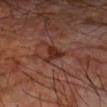<case>
  <biopsy_status>not biopsied; imaged during a skin examination</biopsy_status>
  <lesion_size>
    <long_diameter_mm_approx>2.5</long_diameter_mm_approx>
  </lesion_size>
  <patient>
    <sex>male</sex>
    <age_approx>70</age_approx>
  </patient>
  <site>right forearm</site>
  <lighting>cross-polarized</lighting>
  <automated_metrics>
    <area_mm2_approx>4.5</area_mm2_approx>
    <shape_asymmetry>0.55</shape_asymmetry>
    <border_irregularity_0_10>5.0</border_irregularity_0_10>
    <color_variation_0_10>2.5</color_variation_0_10>
    <peripheral_color_asymmetry>1.0</peripheral_color_asymmetry>
    <nevus_likeness_0_100>10</nevus_likeness_0_100>
    <lesion_detection_confidence_0_100>100</lesion_detection_confidence_0_100>
  </automated_metrics>
  <image>
    <source>total-body photography crop</source>
    <field_of_view_mm>15</field_of_view_mm>
  </image>
</case>